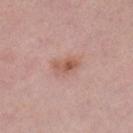Clinical impression: Part of a total-body skin-imaging series; this lesion was reviewed on a skin check and was not flagged for biopsy. Background: A 15 mm crop from a total-body photograph taken for skin-cancer surveillance. Measured at roughly 3.5 mm in maximum diameter. From the left lower leg. Captured under white-light illumination. A female subject, aged approximately 40.